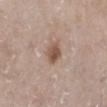follow-up — imaged on a skin check; not biopsied
image-analysis metrics — a lesion area of about 5 mm², a shape eccentricity near 0.55, and a shape-asymmetry score of about 0.2 (0 = symmetric); a mean CIELAB color near L≈52 a*≈18 b*≈26, roughly 12 lightness units darker than nearby skin, and a normalized border contrast of about 8.5; a border-irregularity index near 1.5/10, a within-lesion color-variation index near 3.5/10, and radial color variation of about 1.5; a classifier nevus-likeness of about 85/100
subject — female, aged 63 to 67
tile lighting — white-light illumination
location — the right lower leg
image — total-body-photography crop, ~15 mm field of view
size — ≈2.5 mm